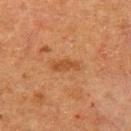Cropped from a whole-body photographic skin survey; the tile spans about 15 mm.
This is a cross-polarized tile.
Approximately 3.5 mm at its widest.
The subject is a female roughly 50 years of age.
The lesion is located on the left thigh.
The total-body-photography lesion software estimated a lesion area of about 4.5 mm², an eccentricity of roughly 0.9, and a shape-asymmetry score of about 0.3 (0 = symmetric). And it measured an average lesion color of about L≈41 a*≈22 b*≈34 (CIELAB) and a lesion-to-skin contrast of about 6 (normalized; higher = more distinct).